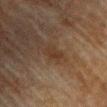No biopsy was performed on this lesion — it was imaged during a full skin examination and was not determined to be concerning. A 15 mm close-up tile from a total-body photography series done for melanoma screening. The subject is a male aged approximately 75. The lesion is on the chest.